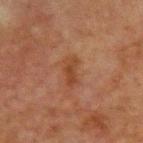biopsy status: catalogued during a skin exam; not biopsied
acquisition: ~15 mm crop, total-body skin-cancer survey
automated metrics: a footprint of about 4.5 mm², an eccentricity of roughly 0.9, and a symmetry-axis asymmetry near 0.3; a mean CIELAB color near L≈34 a*≈20 b*≈29, roughly 6 lightness units darker than nearby skin, and a lesion-to-skin contrast of about 7 (normalized; higher = more distinct)
site: the front of the torso
tile lighting: cross-polarized
subject: male, aged 63 to 67